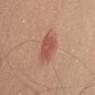{"biopsy_status": "not biopsied; imaged during a skin examination", "image": {"source": "total-body photography crop", "field_of_view_mm": 15}, "lesion_size": {"long_diameter_mm_approx": 3.5}, "site": "arm", "automated_metrics": {"cielab_L": 53, "cielab_a": 26, "cielab_b": 29, "vs_skin_darker_L": 10.0, "vs_skin_contrast_norm": 7.0, "border_irregularity_0_10": 1.5, "color_variation_0_10": 3.0, "peripheral_color_asymmetry": 1.0, "nevus_likeness_0_100": 100, "lesion_detection_confidence_0_100": 100}, "lighting": "white-light", "patient": {"sex": "male", "age_approx": 55}}